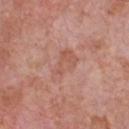The lesion was tiled from a total-body skin photograph and was not biopsied. This is a white-light tile. A male subject, in their 60s. Located on the chest. A 15 mm crop from a total-body photograph taken for skin-cancer surveillance. The recorded lesion diameter is about 4 mm.